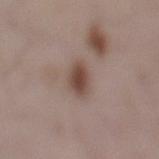Captured during whole-body skin photography for melanoma surveillance; the lesion was not biopsied. A female patient, aged approximately 30. A close-up tile cropped from a whole-body skin photograph, about 15 mm across. The lesion's longest dimension is about 3 mm. The total-body-photography lesion software estimated a lesion area of about 5.5 mm². On the left lower leg.